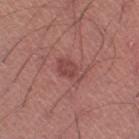Assessment: Part of a total-body skin-imaging series; this lesion was reviewed on a skin check and was not flagged for biopsy. Acquisition and patient details: This is a white-light tile. The lesion is on the left thigh. A male subject, approximately 45 years of age. The recorded lesion diameter is about 3.5 mm. A 15 mm close-up tile from a total-body photography series done for melanoma screening. Automated tile analysis of the lesion measured a lesion area of about 6 mm², an eccentricity of roughly 0.85, and a symmetry-axis asymmetry near 0.3. The software also gave roughly 8 lightness units darker than nearby skin and a normalized lesion–skin contrast near 6. And it measured border irregularity of about 3 on a 0–10 scale, a color-variation rating of about 2.5/10, and radial color variation of about 1.